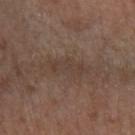Part of a total-body skin-imaging series; this lesion was reviewed on a skin check and was not flagged for biopsy.